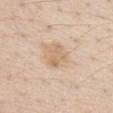acquisition: total-body-photography crop, ~15 mm field of view
location: the chest
subject: female, aged 58–62
image-analysis metrics: a lesion area of about 6.5 mm², an eccentricity of roughly 0.55, and a symmetry-axis asymmetry near 0.25; a lesion color around L≈67 a*≈16 b*≈33 in CIELAB, a lesion–skin lightness drop of about 8, and a lesion-to-skin contrast of about 5.5 (normalized; higher = more distinct)
lesion diameter: ~3.5 mm (longest diameter)